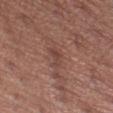  automated_metrics:
    area_mm2_approx: 3.5
    eccentricity: 0.85
    shape_asymmetry: 0.4
    border_irregularity_0_10: 4.0
    peripheral_color_asymmetry: 0.0
    nevus_likeness_0_100: 0
    lesion_detection_confidence_0_100: 80
  patient:
    sex: female
    age_approx: 30
  lesion_size:
    long_diameter_mm_approx: 3.0
  site: abdomen
  lighting: white-light
  image:
    source: total-body photography crop
    field_of_view_mm: 15